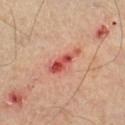  biopsy_status: not biopsied; imaged during a skin examination
  site: leg
  patient:
    sex: male
    age_approx: 65
  image:
    source: total-body photography crop
    field_of_view_mm: 15
  lesion_size:
    long_diameter_mm_approx: 4.5
  automated_metrics:
    vs_skin_darker_L: 14.0
    vs_skin_contrast_norm: 9.0
    nevus_likeness_0_100: 0
    lesion_detection_confidence_0_100: 100
  lighting: cross-polarized